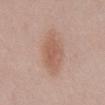Notes:
- notes · catalogued during a skin exam; not biopsied
- patient · male, roughly 55 years of age
- lesion size · about 6.5 mm
- image · ~15 mm crop, total-body skin-cancer survey
- location · the abdomen
- illumination · white-light illumination